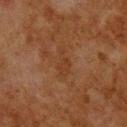Captured during whole-body skin photography for melanoma surveillance; the lesion was not biopsied.
A 15 mm close-up tile from a total-body photography series done for melanoma screening.
A male subject approximately 80 years of age.
Measured at roughly 3.5 mm in maximum diameter.
Automated tile analysis of the lesion measured a footprint of about 4.5 mm² and a shape eccentricity near 0.9. It also reported a border-irregularity index near 4.5/10, a color-variation rating of about 1/10, and a peripheral color-asymmetry measure near 0.5.
Imaged with cross-polarized lighting.
The lesion is located on the upper back.